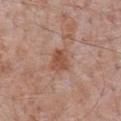workup = no biopsy performed (imaged during a skin exam)
imaging modality = 15 mm crop, total-body photography
lesion diameter = about 3 mm
image-analysis metrics = a mean CIELAB color near L≈51 a*≈22 b*≈29 and about 9 CIELAB-L* units darker than the surrounding skin; border irregularity of about 2.5 on a 0–10 scale, a within-lesion color-variation index near 3/10, and a peripheral color-asymmetry measure near 1; lesion-presence confidence of about 100/100
subject = male, aged approximately 70
anatomic site = the chest
tile lighting = white-light